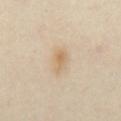Q: How was the tile lit?
A: cross-polarized illumination
Q: Automated lesion metrics?
A: an area of roughly 5.5 mm², an outline eccentricity of about 0.8 (0 = round, 1 = elongated), and a shape-asymmetry score of about 0.15 (0 = symmetric); a border-irregularity rating of about 1.5/10, a color-variation rating of about 3.5/10, and radial color variation of about 1
Q: What is the anatomic site?
A: the front of the torso
Q: Lesion size?
A: about 3.5 mm
Q: Who is the patient?
A: female, about 40 years old
Q: What kind of image is this?
A: ~15 mm crop, total-body skin-cancer survey